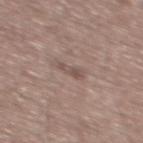<record>
  <biopsy_status>not biopsied; imaged during a skin examination</biopsy_status>
  <patient>
    <sex>male</sex>
    <age_approx>60</age_approx>
  </patient>
  <lesion_size>
    <long_diameter_mm_approx>2.5</long_diameter_mm_approx>
  </lesion_size>
  <site>upper back</site>
  <image>
    <source>total-body photography crop</source>
    <field_of_view_mm>15</field_of_view_mm>
  </image>
  <lighting>white-light</lighting>
  <automated_metrics>
    <cielab_L>50</cielab_L>
    <cielab_a>15</cielab_a>
    <cielab_b>21</cielab_b>
    <vs_skin_darker_L>8.0</vs_skin_darker_L>
    <vs_skin_contrast_norm>6.0</vs_skin_contrast_norm>
  </automated_metrics>
</record>